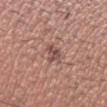Notes:
* lighting: white-light illumination
* image: 15 mm crop, total-body photography
* site: the head or neck
* subject: male, approximately 40 years of age